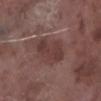| key | value |
|---|---|
| notes | imaged on a skin check; not biopsied |
| acquisition | ~15 mm crop, total-body skin-cancer survey |
| lighting | white-light |
| lesion diameter | ≈5 mm |
| TBP lesion metrics | a lesion color around L≈37 a*≈19 b*≈20 in CIELAB, a lesion–skin lightness drop of about 8, and a normalized lesion–skin contrast near 7; a within-lesion color-variation index near 2.5/10 and a peripheral color-asymmetry measure near 1; a nevus-likeness score of about 0/100 and a lesion-detection confidence of about 100/100 |
| subject | male, aged 73–77 |
| location | the right lower leg |